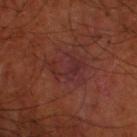This lesion was catalogued during total-body skin photography and was not selected for biopsy. A male patient approximately 70 years of age. Captured under cross-polarized illumination. The total-body-photography lesion software estimated an area of roughly 10 mm² and a symmetry-axis asymmetry near 0.6. It also reported roughly 4 lightness units darker than nearby skin and a normalized lesion–skin contrast near 6. It also reported a border-irregularity index near 8.5/10, internal color variation of about 2.5 on a 0–10 scale, and radial color variation of about 1. And it measured a classifier nevus-likeness of about 0/100 and a detector confidence of about 100 out of 100 that the crop contains a lesion. On the left lower leg. A 15 mm close-up extracted from a 3D total-body photography capture.